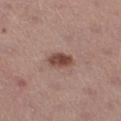Part of a total-body skin-imaging series; this lesion was reviewed on a skin check and was not flagged for biopsy.